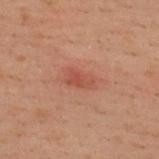<record>
  <biopsy_status>not biopsied; imaged during a skin examination</biopsy_status>
  <lesion_size>
    <long_diameter_mm_approx>3.0</long_diameter_mm_approx>
  </lesion_size>
  <site>upper back</site>
  <lighting>cross-polarized</lighting>
  <patient>
    <sex>male</sex>
    <age_approx>40</age_approx>
  </patient>
  <image>
    <source>total-body photography crop</source>
    <field_of_view_mm>15</field_of_view_mm>
  </image>
</record>